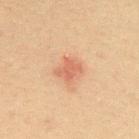This is a cross-polarized tile.
The total-body-photography lesion software estimated an average lesion color of about L≈53 a*≈23 b*≈30 (CIELAB), about 8 CIELAB-L* units darker than the surrounding skin, and a normalized lesion–skin contrast near 6. And it measured a classifier nevus-likeness of about 20/100.
A female patient, approximately 40 years of age.
The lesion's longest dimension is about 3 mm.
Cropped from a total-body skin-imaging series; the visible field is about 15 mm.
Located on the upper back.